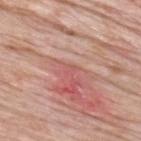Notes:
* tile lighting: white-light
* subject: male, aged around 60
* acquisition: ~15 mm tile from a whole-body skin photo
* image-analysis metrics: two-axis asymmetry of about 0.3; a lesion color around L≈57 a*≈29 b*≈25 in CIELAB, roughly 6 lightness units darker than nearby skin, and a normalized border contrast of about 4; a color-variation rating of about 0/10
* lesion diameter: ≈1.5 mm
* location: the upper back
* histopathology: a nodular basal cell carcinoma — a malignant skin lesion